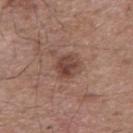The lesion-visualizer software estimated an outline eccentricity of about 0.6 (0 = round, 1 = elongated). The software also gave a nevus-likeness score of about 40/100 and a lesion-detection confidence of about 100/100. About 3 mm across. The lesion is located on the mid back. The patient is a male in their mid- to late 50s. This image is a 15 mm lesion crop taken from a total-body photograph.